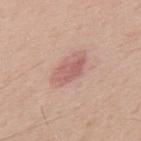Q: Was a biopsy performed?
A: catalogued during a skin exam; not biopsied
Q: Patient demographics?
A: male, roughly 70 years of age
Q: What kind of image is this?
A: ~15 mm tile from a whole-body skin photo
Q: Lesion location?
A: the back